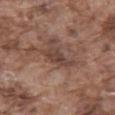Acquisition and patient details:
This is a white-light tile. On the mid back. Cropped from a whole-body photographic skin survey; the tile spans about 15 mm. Approximately 3.5 mm at its widest. A male subject, roughly 75 years of age.